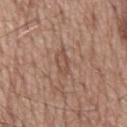Part of a total-body skin-imaging series; this lesion was reviewed on a skin check and was not flagged for biopsy. The tile uses white-light illumination. The lesion is located on the mid back. A male patient, roughly 60 years of age. The recorded lesion diameter is about 3.5 mm. A close-up tile cropped from a whole-body skin photograph, about 15 mm across.